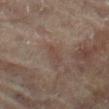The lesion was photographed on a routine skin check and not biopsied; there is no pathology result. The recorded lesion diameter is about 2.5 mm. This is a cross-polarized tile. The total-body-photography lesion software estimated a mean CIELAB color near L≈39 a*≈15 b*≈21 and about 5 CIELAB-L* units darker than the surrounding skin. The software also gave a border-irregularity rating of about 4/10, internal color variation of about 0 on a 0–10 scale, and radial color variation of about 0. Located on the leg. This image is a 15 mm lesion crop taken from a total-body photograph. A female subject, aged around 80.